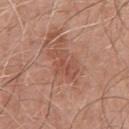biopsy status = total-body-photography surveillance lesion; no biopsy | anatomic site = the chest | patient = male, aged around 50 | imaging modality = ~15 mm crop, total-body skin-cancer survey.